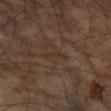Clinical impression:
Recorded during total-body skin imaging; not selected for excision or biopsy.